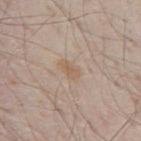Part of a total-body skin-imaging series; this lesion was reviewed on a skin check and was not flagged for biopsy. Cropped from a total-body skin-imaging series; the visible field is about 15 mm. The tile uses white-light illumination. Approximately 3 mm at its widest. A male subject, aged 78–82. The lesion is located on the mid back.